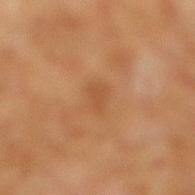The lesion was tiled from a total-body skin photograph and was not biopsied. A male subject aged 58 to 62. This image is a 15 mm lesion crop taken from a total-body photograph. On the right lower leg.